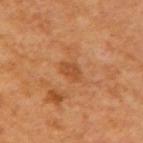A 15 mm close-up extracted from a 3D total-body photography capture.
The lesion is on the arm.
A female subject in their mid-50s.